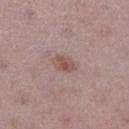Case summary:
- follow-up · no biopsy performed (imaged during a skin exam)
- illumination · white-light illumination
- diameter · about 3 mm
- automated metrics · an average lesion color of about L≈52 a*≈18 b*≈22 (CIELAB) and roughly 9 lightness units darker than nearby skin; a nevus-likeness score of about 35/100 and lesion-presence confidence of about 100/100
- subject · female, in their mid-40s
- location · the right lower leg
- acquisition · total-body-photography crop, ~15 mm field of view